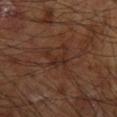Impression:
The lesion was photographed on a routine skin check and not biopsied; there is no pathology result.
Clinical summary:
A male patient aged 63 to 67. From the right forearm. A region of skin cropped from a whole-body photographic capture, roughly 15 mm wide. Imaged with cross-polarized lighting.